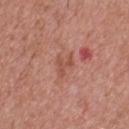Background:
A male patient aged approximately 65. Cropped from a whole-body photographic skin survey; the tile spans about 15 mm. The lesion-visualizer software estimated an outline eccentricity of about 0.8 (0 = round, 1 = elongated) and a symmetry-axis asymmetry near 0.65. The software also gave an automated nevus-likeness rating near 0 out of 100 and a lesion-detection confidence of about 100/100. This is a white-light tile. The lesion is on the upper back.